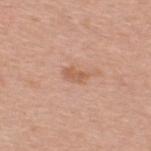| key | value |
|---|---|
| notes | total-body-photography surveillance lesion; no biopsy |